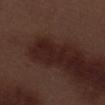Clinical summary: Imaged with white-light lighting. A roughly 15 mm field-of-view crop from a total-body skin photograph. About 4.5 mm across. Located on the left thigh. The subject is a male aged 68–72.